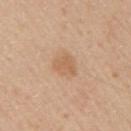Recorded during total-body skin imaging; not selected for excision or biopsy. This is a white-light tile. An algorithmic analysis of the crop reported a border-irregularity rating of about 2.5/10, internal color variation of about 1.5 on a 0–10 scale, and radial color variation of about 0.5. It also reported a classifier nevus-likeness of about 40/100 and a lesion-detection confidence of about 100/100. On the right upper arm. A male patient aged 33–37. A 15 mm close-up extracted from a 3D total-body photography capture. Longest diameter approximately 3 mm.